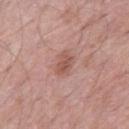Findings:
– biopsy status: imaged on a skin check; not biopsied
– imaging modality: total-body-photography crop, ~15 mm field of view
– automated lesion analysis: a lesion area of about 5 mm², an outline eccentricity of about 0.75 (0 = round, 1 = elongated), and a shape-asymmetry score of about 0.25 (0 = symmetric); a within-lesion color-variation index near 2.5/10 and a peripheral color-asymmetry measure near 1; a lesion-detection confidence of about 100/100
– subject: male, aged 53–57
– body site: the right thigh
– lighting: white-light illumination